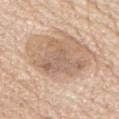notes: imaged on a skin check; not biopsied | patient: male, roughly 75 years of age | image: total-body-photography crop, ~15 mm field of view | body site: the chest | size: ≈5.5 mm | automated metrics: a footprint of about 16 mm², an outline eccentricity of about 0.6 (0 = round, 1 = elongated), and a symmetry-axis asymmetry near 0.55; a border-irregularity index near 6.5/10 and internal color variation of about 4 on a 0–10 scale | illumination: white-light.